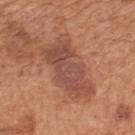Assessment: This lesion was catalogued during total-body skin photography and was not selected for biopsy. Acquisition and patient details: Approximately 7.5 mm at its widest. Located on the back. Automated image analysis of the tile measured an eccentricity of roughly 0.85. The software also gave an average lesion color of about L≈49 a*≈24 b*≈29 (CIELAB), roughly 9 lightness units darker than nearby skin, and a normalized lesion–skin contrast near 7. The analysis additionally found a color-variation rating of about 4/10 and a peripheral color-asymmetry measure near 1. It also reported a lesion-detection confidence of about 100/100. A 15 mm crop from a total-body photograph taken for skin-cancer surveillance. A male subject approximately 65 years of age. The tile uses white-light illumination.